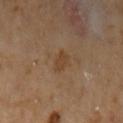Clinical impression: The lesion was tiled from a total-body skin photograph and was not biopsied. Clinical summary: A region of skin cropped from a whole-body photographic capture, roughly 15 mm wide. The patient is a female aged 58 to 62. About 3 mm across. This is a cross-polarized tile. The total-body-photography lesion software estimated an average lesion color of about L≈41 a*≈17 b*≈31 (CIELAB) and a normalized border contrast of about 6. It also reported a nevus-likeness score of about 10/100 and lesion-presence confidence of about 100/100. From the arm.